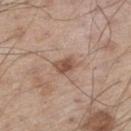Assessment:
Recorded during total-body skin imaging; not selected for excision or biopsy.
Background:
Cropped from a whole-body photographic skin survey; the tile spans about 15 mm. A male patient, about 60 years old. The lesion is located on the left thigh.